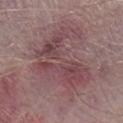Assessment:
The lesion was tiled from a total-body skin photograph and was not biopsied.
Clinical summary:
The recorded lesion diameter is about 8 mm. A male subject, approximately 75 years of age. The lesion is on the right lower leg. Cropped from a total-body skin-imaging series; the visible field is about 15 mm. Captured under white-light illumination.